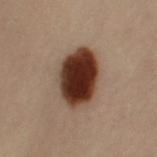{"biopsy_status": "not biopsied; imaged during a skin examination", "lesion_size": {"long_diameter_mm_approx": 6.5}, "image": {"source": "total-body photography crop", "field_of_view_mm": 15}, "lighting": "cross-polarized", "automated_metrics": {"area_mm2_approx": 22.0, "eccentricity": 0.7, "shape_asymmetry": 0.1, "color_variation_0_10": 7.0, "peripheral_color_asymmetry": 2.0}, "patient": {"sex": "female", "age_approx": 60}, "site": "leg"}